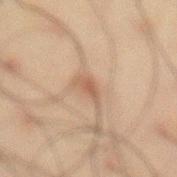| feature | finding |
|---|---|
| follow-up | catalogued during a skin exam; not biopsied |